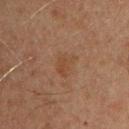Clinical impression: Recorded during total-body skin imaging; not selected for excision or biopsy. Background: A male patient, aged 43 to 47. Automated image analysis of the tile measured a lesion area of about 5 mm² and an outline eccentricity of about 0.7 (0 = round, 1 = elongated). It also reported a lesion color around L≈36 a*≈17 b*≈26 in CIELAB, roughly 4 lightness units darker than nearby skin, and a lesion-to-skin contrast of about 4.5 (normalized; higher = more distinct). It also reported an automated nevus-likeness rating near 5 out of 100 and a detector confidence of about 100 out of 100 that the crop contains a lesion. A 15 mm crop from a total-body photograph taken for skin-cancer surveillance. The tile uses cross-polarized illumination. Located on the chest.